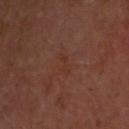notes = imaged on a skin check; not biopsied | site = the head or neck | image = ~15 mm tile from a whole-body skin photo | patient = male, about 60 years old | TBP lesion metrics = a shape eccentricity near 0.9 and a symmetry-axis asymmetry near 0.35; an average lesion color of about L≈31 a*≈20 b*≈26 (CIELAB) and a lesion–skin lightness drop of about 3; an automated nevus-likeness rating near 0 out of 100 and lesion-presence confidence of about 100/100 | size = ≈3 mm | lighting = cross-polarized.